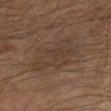biopsy_status: not biopsied; imaged during a skin examination
site: right lower leg
lesion_size:
  long_diameter_mm_approx: 6.5
automated_metrics:
  area_mm2_approx: 18.0
  eccentricity: 0.8
  cielab_L: 36
  cielab_a: 14
  cielab_b: 23
  vs_skin_darker_L: 5.0
  vs_skin_contrast_norm: 4.5
  border_irregularity_0_10: 5.5
  peripheral_color_asymmetry: 1.5
lighting: cross-polarized
image:
  source: total-body photography crop
  field_of_view_mm: 15
patient:
  sex: male
  age_approx: 65